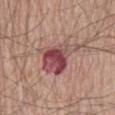* notes — total-body-photography surveillance lesion; no biopsy
* patient — male, about 80 years old
* automated lesion analysis — a lesion area of about 14 mm² and an outline eccentricity of about 0.75 (0 = round, 1 = elongated); an average lesion color of about L≈48 a*≈26 b*≈20 (CIELAB), roughly 13 lightness units darker than nearby skin, and a normalized lesion–skin contrast near 10; a border-irregularity index near 5/10 and radial color variation of about 3.5
* body site — the abdomen
* image source — ~15 mm tile from a whole-body skin photo
* illumination — white-light